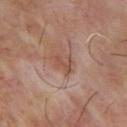{"biopsy_status": "not biopsied; imaged during a skin examination", "site": "upper back", "automated_metrics": {"area_mm2_approx": 3.0, "shape_asymmetry": 0.4, "cielab_L": 46, "cielab_a": 19, "cielab_b": 26, "vs_skin_darker_L": 7.0, "vs_skin_contrast_norm": 6.5, "border_irregularity_0_10": 5.5, "color_variation_0_10": 0.0, "peripheral_color_asymmetry": 0.0, "nevus_likeness_0_100": 0}, "patient": {"sex": "male", "age_approx": 65}, "image": {"source": "total-body photography crop", "field_of_view_mm": 15}}